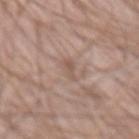biopsy status: imaged on a skin check; not biopsied | subject: male, approximately 65 years of age | image: ~15 mm tile from a whole-body skin photo | automated lesion analysis: a classifier nevus-likeness of about 0/100 and lesion-presence confidence of about 90/100 | size: ~2.5 mm (longest diameter) | lighting: white-light illumination | anatomic site: the abdomen.